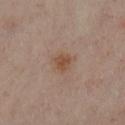The lesion was photographed on a routine skin check and not biopsied; there is no pathology result.
A region of skin cropped from a whole-body photographic capture, roughly 15 mm wide.
Captured under cross-polarized illumination.
An algorithmic analysis of the crop reported a border-irregularity rating of about 2.5/10, a within-lesion color-variation index near 3/10, and radial color variation of about 1. The analysis additionally found a nevus-likeness score of about 70/100.
A female subject, aged around 55.
About 3 mm across.
The lesion is on the left lower leg.